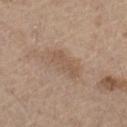Q: Is there a histopathology result?
A: no biopsy performed (imaged during a skin exam)
Q: Lesion size?
A: ~4 mm (longest diameter)
Q: What lighting was used for the tile?
A: white-light illumination
Q: What kind of image is this?
A: ~15 mm crop, total-body skin-cancer survey
Q: Lesion location?
A: the left thigh
Q: Patient demographics?
A: male, aged approximately 70